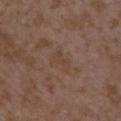workup: no biopsy performed (imaged during a skin exam)
lighting: white-light illumination
image-analysis metrics: a shape eccentricity near 0.85 and a shape-asymmetry score of about 0.45 (0 = symmetric); border irregularity of about 4.5 on a 0–10 scale, a within-lesion color-variation index near 0.5/10, and radial color variation of about 0; a nevus-likeness score of about 0/100
body site: the left upper arm
subject: female, roughly 35 years of age
acquisition: total-body-photography crop, ~15 mm field of view